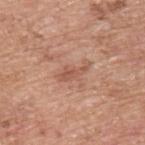The lesion was photographed on a routine skin check and not biopsied; there is no pathology result.
A close-up tile cropped from a whole-body skin photograph, about 15 mm across.
An algorithmic analysis of the crop reported a border-irregularity index near 4/10 and a peripheral color-asymmetry measure near 1. The analysis additionally found a classifier nevus-likeness of about 0/100 and a detector confidence of about 100 out of 100 that the crop contains a lesion.
The patient is a male roughly 55 years of age.
Located on the upper back.